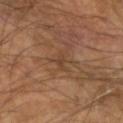{
  "image": {
    "source": "total-body photography crop",
    "field_of_view_mm": 15
  },
  "site": "left forearm",
  "patient": {
    "sex": "male",
    "age_approx": 65
  },
  "lighting": "cross-polarized",
  "lesion_size": {
    "long_diameter_mm_approx": 3.0
  }
}